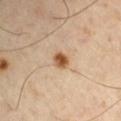{
  "image": {
    "source": "total-body photography crop",
    "field_of_view_mm": 15
  },
  "site": "arm",
  "lighting": "cross-polarized",
  "patient": {
    "sex": "male",
    "age_approx": 65
  },
  "lesion_size": {
    "long_diameter_mm_approx": 2.0
  }
}